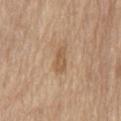biopsy status: catalogued during a skin exam; not biopsied
automated metrics: a lesion color around L≈56 a*≈17 b*≈34 in CIELAB, a lesion–skin lightness drop of about 9, and a normalized border contrast of about 6.5
diameter: about 3 mm
site: the back
image source: ~15 mm crop, total-body skin-cancer survey
illumination: white-light illumination
patient: male, aged approximately 70